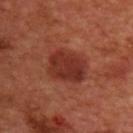Background: About 4.5 mm across. The tile uses cross-polarized illumination. A lesion tile, about 15 mm wide, cut from a 3D total-body photograph. From the upper back. The patient is a male about 50 years old.